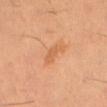No biopsy was performed on this lesion — it was imaged during a full skin examination and was not determined to be concerning.
A 15 mm crop from a total-body photograph taken for skin-cancer surveillance.
The lesion is on the left thigh.
A male patient roughly 60 years of age.
This is a cross-polarized tile.
The lesion's longest dimension is about 4 mm.